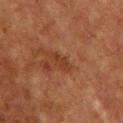Q: Was a biopsy performed?
A: catalogued during a skin exam; not biopsied
Q: How was this image acquired?
A: ~15 mm tile from a whole-body skin photo
Q: Who is the patient?
A: male, about 60 years old
Q: Lesion location?
A: the chest